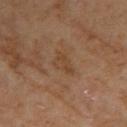Notes:
- follow-up — no biopsy performed (imaged during a skin exam)
- subject — female, approximately 60 years of age
- lesion size — ~3.5 mm (longest diameter)
- automated lesion analysis — a footprint of about 5 mm², an outline eccentricity of about 0.8 (0 = round, 1 = elongated), and a shape-asymmetry score of about 0.4 (0 = symmetric)
- illumination — cross-polarized
- image — ~15 mm crop, total-body skin-cancer survey
- anatomic site — the upper back